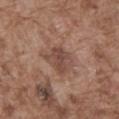Clinical impression:
The lesion was photographed on a routine skin check and not biopsied; there is no pathology result.
Background:
A 15 mm close-up tile from a total-body photography series done for melanoma screening. A male patient aged 73–77. From the front of the torso. Measured at roughly 3.5 mm in maximum diameter.